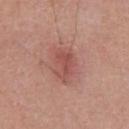<record>
  <site>chest</site>
  <patient>
    <sex>male</sex>
    <age_approx>55</age_approx>
  </patient>
  <image>
    <source>total-body photography crop</source>
    <field_of_view_mm>15</field_of_view_mm>
  </image>
</record>